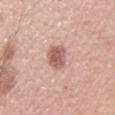On the left upper arm. A female subject, aged approximately 50. A 15 mm crop from a total-body photograph taken for skin-cancer surveillance.